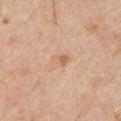A 15 mm crop from a total-body photograph taken for skin-cancer surveillance.
A male patient aged 53–57.
Captured under white-light illumination.
Measured at roughly 2.5 mm in maximum diameter.
From the right upper arm.